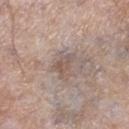biopsy status: catalogued during a skin exam; not biopsied
imaging modality: ~15 mm crop, total-body skin-cancer survey
site: the right lower leg
subject: male, aged approximately 75
automated lesion analysis: a footprint of about 3.5 mm², an outline eccentricity of about 0.75 (0 = round, 1 = elongated), and two-axis asymmetry of about 0.4; a nevus-likeness score of about 5/100 and a detector confidence of about 65 out of 100 that the crop contains a lesion
lighting: white-light